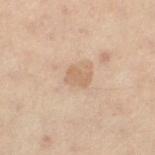Notes:
- notes — catalogued during a skin exam; not biopsied
- lighting — cross-polarized
- patient — female, in their 30s
- size — ~2.5 mm (longest diameter)
- acquisition — ~15 mm tile from a whole-body skin photo
- anatomic site — the left thigh